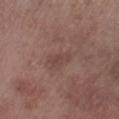Clinical impression:
Part of a total-body skin-imaging series; this lesion was reviewed on a skin check and was not flagged for biopsy.
Image and clinical context:
This image is a 15 mm lesion crop taken from a total-body photograph. Captured under white-light illumination. Approximately 3 mm at its widest. Automated image analysis of the tile measured a lesion area of about 4.5 mm² and a shape-asymmetry score of about 0.3 (0 = symmetric). It also reported a lesion color around L≈44 a*≈18 b*≈22 in CIELAB and a normalized lesion–skin contrast near 5. The lesion is on the right lower leg. A male subject aged 53 to 57.